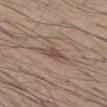Imaged during a routine full-body skin examination; the lesion was not biopsied and no histopathology is available. On the abdomen. A lesion tile, about 15 mm wide, cut from a 3D total-body photograph. The recorded lesion diameter is about 2.5 mm. The patient is a male aged approximately 40. This is a white-light tile. Automated tile analysis of the lesion measured a lesion area of about 3.5 mm², an outline eccentricity of about 0.8 (0 = round, 1 = elongated), and a shape-asymmetry score of about 0.25 (0 = symmetric). It also reported a lesion–skin lightness drop of about 9 and a lesion-to-skin contrast of about 6.5 (normalized; higher = more distinct). It also reported a classifier nevus-likeness of about 0/100 and lesion-presence confidence of about 90/100.